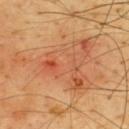Impression:
Part of a total-body skin-imaging series; this lesion was reviewed on a skin check and was not flagged for biopsy.
Context:
Imaged with cross-polarized lighting. A 15 mm close-up extracted from a 3D total-body photography capture. The subject is a male aged 63 to 67. About 10.5 mm across. The lesion is on the upper back.